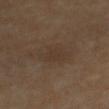Clinical summary: The lesion is on the chest. The patient is a female roughly 65 years of age. The tile uses cross-polarized illumination. Cropped from a total-body skin-imaging series; the visible field is about 15 mm.